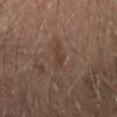Impression: Captured during whole-body skin photography for melanoma surveillance; the lesion was not biopsied. Acquisition and patient details: Measured at roughly 2.5 mm in maximum diameter. The lesion is located on the right forearm. A 15 mm close-up extracted from a 3D total-body photography capture. Imaged with cross-polarized lighting. A male subject in their mid- to late 60s. Automated tile analysis of the lesion measured a footprint of about 2.5 mm², an outline eccentricity of about 0.9 (0 = round, 1 = elongated), and a shape-asymmetry score of about 0.5 (0 = symmetric). The analysis additionally found border irregularity of about 5 on a 0–10 scale and internal color variation of about 0 on a 0–10 scale. The analysis additionally found a nevus-likeness score of about 0/100 and lesion-presence confidence of about 95/100.